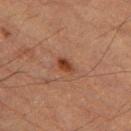Imaged during a routine full-body skin examination; the lesion was not biopsied and no histopathology is available.